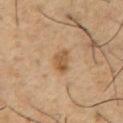The lesion was tiled from a total-body skin photograph and was not biopsied.
On the chest.
The recorded lesion diameter is about 3 mm.
Cropped from a whole-body photographic skin survey; the tile spans about 15 mm.
The subject is a male aged 53–57.
Captured under cross-polarized illumination.